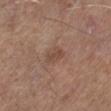Clinical impression: Imaged during a routine full-body skin examination; the lesion was not biopsied and no histopathology is available. Acquisition and patient details: The recorded lesion diameter is about 2.5 mm. From the left lower leg. A lesion tile, about 15 mm wide, cut from a 3D total-body photograph. The subject is a male about 65 years old. Captured under white-light illumination.